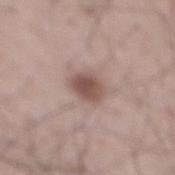Assessment:
The lesion was tiled from a total-body skin photograph and was not biopsied.
Clinical summary:
An algorithmic analysis of the crop reported a footprint of about 6.5 mm², a shape eccentricity near 0.6, and a shape-asymmetry score of about 0.15 (0 = symmetric). The analysis additionally found a lesion color around L≈51 a*≈18 b*≈22 in CIELAB and a lesion–skin lightness drop of about 12. The software also gave a lesion-detection confidence of about 100/100. A 15 mm close-up tile from a total-body photography series done for melanoma screening. Imaged with white-light lighting. The subject is a male in their mid- to late 60s. The recorded lesion diameter is about 3 mm. Located on the mid back.